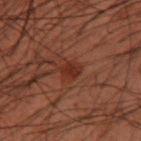Part of a total-body skin-imaging series; this lesion was reviewed on a skin check and was not flagged for biopsy. This is a cross-polarized tile. Measured at roughly 2.5 mm in maximum diameter. A male subject, approximately 60 years of age. The lesion is located on the right forearm. Cropped from a total-body skin-imaging series; the visible field is about 15 mm.